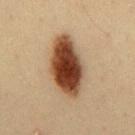<lesion>
<biopsy_status>not biopsied; imaged during a skin examination</biopsy_status>
<site>mid back</site>
<lesion_size>
  <long_diameter_mm_approx>7.5</long_diameter_mm_approx>
</lesion_size>
<automated_metrics>
  <cielab_L>39</cielab_L>
  <cielab_a>19</cielab_a>
  <cielab_b>30</cielab_b>
  <vs_skin_contrast_norm>15.0</vs_skin_contrast_norm>
  <border_irregularity_0_10>1.5</border_irregularity_0_10>
  <color_variation_0_10>7.5</color_variation_0_10>
  <lesion_detection_confidence_0_100>100</lesion_detection_confidence_0_100>
</automated_metrics>
<lighting>cross-polarized</lighting>
<image>
  <source>total-body photography crop</source>
  <field_of_view_mm>15</field_of_view_mm>
</image>
<patient>
  <sex>male</sex>
  <age_approx>35</age_approx>
</patient>
</lesion>